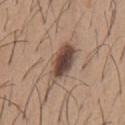Impression: This lesion was catalogued during total-body skin photography and was not selected for biopsy. Context: Imaged with white-light lighting. A roughly 15 mm field-of-view crop from a total-body skin photograph. On the chest. About 6 mm across. A male subject, approximately 65 years of age.